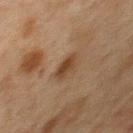Notes:
* follow-up · total-body-photography surveillance lesion; no biopsy
* imaging modality · ~15 mm crop, total-body skin-cancer survey
* subject · male, in their mid-70s
* site · the chest